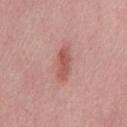{"biopsy_status": "not biopsied; imaged during a skin examination", "lesion_size": {"long_diameter_mm_approx": 4.0}, "site": "front of the torso", "patient": {"sex": "male", "age_approx": 30}, "automated_metrics": {"area_mm2_approx": 7.0, "eccentricity": 0.9, "cielab_L": 56, "cielab_a": 26, "cielab_b": 26, "vs_skin_contrast_norm": 7.0}, "lighting": "white-light", "image": {"source": "total-body photography crop", "field_of_view_mm": 15}}